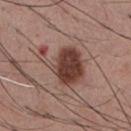Assessment: The lesion was tiled from a total-body skin photograph and was not biopsied. Clinical summary: A close-up tile cropped from a whole-body skin photograph, about 15 mm across. A male subject aged 53 to 57. From the chest.